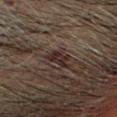The lesion was tiled from a total-body skin photograph and was not biopsied.
The tile uses cross-polarized illumination.
A male patient, about 70 years old.
Automated image analysis of the tile measured a mean CIELAB color near L≈20 a*≈13 b*≈14 and about 8 CIELAB-L* units darker than the surrounding skin. The software also gave a nevus-likeness score of about 10/100 and a detector confidence of about 90 out of 100 that the crop contains a lesion.
The lesion's longest dimension is about 3 mm.
Located on the head or neck.
Cropped from a total-body skin-imaging series; the visible field is about 15 mm.